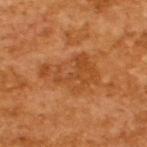Clinical impression: Recorded during total-body skin imaging; not selected for excision or biopsy. Background: A 15 mm close-up tile from a total-body photography series done for melanoma screening. The recorded lesion diameter is about 6 mm. The patient is a male aged 63 to 67.